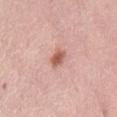Impression: Part of a total-body skin-imaging series; this lesion was reviewed on a skin check and was not flagged for biopsy. Image and clinical context: The lesion is located on the abdomen. Automated image analysis of the tile measured an area of roughly 4 mm² and a symmetry-axis asymmetry near 0.3. The software also gave an average lesion color of about L≈59 a*≈25 b*≈28 (CIELAB), about 12 CIELAB-L* units darker than the surrounding skin, and a lesion-to-skin contrast of about 8.5 (normalized; higher = more distinct). The software also gave a detector confidence of about 100 out of 100 that the crop contains a lesion. Imaged with white-light lighting. Cropped from a whole-body photographic skin survey; the tile spans about 15 mm. The recorded lesion diameter is about 2.5 mm. A female patient, aged 58 to 62.